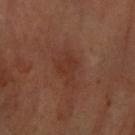- follow-up · catalogued during a skin exam; not biopsied
- lighting · cross-polarized
- acquisition · ~15 mm crop, total-body skin-cancer survey
- patient · approximately 65 years of age
- diameter · about 4 mm
- location · the right forearm